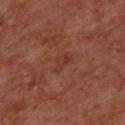No biopsy was performed on this lesion — it was imaged during a full skin examination and was not determined to be concerning. The lesion's longest dimension is about 2.5 mm. A male subject, about 70 years old. The tile uses cross-polarized illumination. Located on the back. A 15 mm close-up extracted from a 3D total-body photography capture.